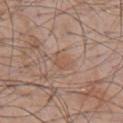Assessment: No biopsy was performed on this lesion — it was imaged during a full skin examination and was not determined to be concerning. Image and clinical context: The total-body-photography lesion software estimated a footprint of about 5 mm², an outline eccentricity of about 0.7 (0 = round, 1 = elongated), and a symmetry-axis asymmetry near 0.2. Imaged with white-light lighting. From the front of the torso. A male subject in their mid-50s. A 15 mm close-up tile from a total-body photography series done for melanoma screening. About 3 mm across.